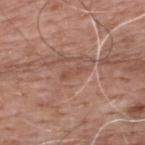workup = imaged on a skin check; not biopsied
acquisition = total-body-photography crop, ~15 mm field of view
patient = male, aged approximately 60
location = the upper back